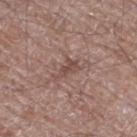Notes:
- follow-up · imaged on a skin check; not biopsied
- patient · male, in their 70s
- body site · the right thigh
- image · total-body-photography crop, ~15 mm field of view
- size · about 2.5 mm
- lighting · white-light